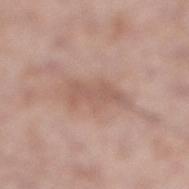Captured during whole-body skin photography for melanoma surveillance; the lesion was not biopsied. Captured under white-light illumination. A close-up tile cropped from a whole-body skin photograph, about 15 mm across. A male subject aged 53 to 57. About 5 mm across. The lesion is located on the leg.